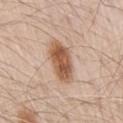Assessment:
This lesion was catalogued during total-body skin photography and was not selected for biopsy.
Context:
A region of skin cropped from a whole-body photographic capture, roughly 15 mm wide. The subject is a male in their 70s. From the right upper arm.